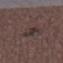Q: What is the anatomic site?
A: the arm
Q: Patient demographics?
A: female, about 50 years old
Q: How was this image acquired?
A: ~15 mm crop, total-body skin-cancer survey
Q: Illumination type?
A: white-light illumination